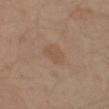| feature | finding |
|---|---|
| notes | total-body-photography surveillance lesion; no biopsy |
| subject | male, about 30 years old |
| diameter | ≈3.5 mm |
| location | the left upper arm |
| image-analysis metrics | an area of roughly 6 mm², an eccentricity of roughly 0.75, and a symmetry-axis asymmetry near 0.2 |
| imaging modality | 15 mm crop, total-body photography |